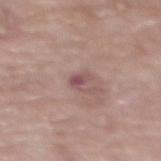Clinical impression:
The lesion was photographed on a routine skin check and not biopsied; there is no pathology result.
Context:
The lesion is located on the chest. This is a white-light tile. Cropped from a total-body skin-imaging series; the visible field is about 15 mm. The patient is a female aged approximately 65.